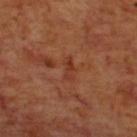Case summary:
• follow-up — no biopsy performed (imaged during a skin exam)
• tile lighting — cross-polarized illumination
• subject — male, approximately 70 years of age
• automated lesion analysis — a mean CIELAB color near L≈35 a*≈26 b*≈31, a lesion–skin lightness drop of about 6, and a lesion-to-skin contrast of about 5.5 (normalized; higher = more distinct); a color-variation rating of about 0/10
• site — the upper back
• imaging modality — total-body-photography crop, ~15 mm field of view